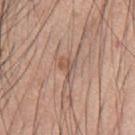follow-up: imaged on a skin check; not biopsied
automated lesion analysis: a footprint of about 3.5 mm², an eccentricity of roughly 0.6, and a symmetry-axis asymmetry near 0.65; a mean CIELAB color near L≈54 a*≈19 b*≈28, a lesion–skin lightness drop of about 8, and a lesion-to-skin contrast of about 6 (normalized; higher = more distinct)
lesion size: about 3 mm
body site: the front of the torso
image: ~15 mm tile from a whole-body skin photo
subject: male, about 60 years old